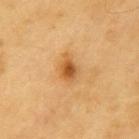image:
  source: total-body photography crop
  field_of_view_mm: 15
patient:
  sex: male
  age_approx: 60
automated_metrics:
  cielab_L: 56
  cielab_a: 24
  cielab_b: 45
  vs_skin_darker_L: 12.0
  vs_skin_contrast_norm: 8.5
  border_irregularity_0_10: 2.5
  peripheral_color_asymmetry: 2.0
  nevus_likeness_0_100: 90
  lesion_detection_confidence_0_100: 100
site: left upper arm
lighting: cross-polarized